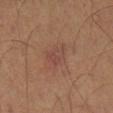The lesion was photographed on a routine skin check and not biopsied; there is no pathology result. About 2.5 mm across. Captured under cross-polarized illumination. A close-up tile cropped from a whole-body skin photograph, about 15 mm across. Automated image analysis of the tile measured a footprint of about 4.5 mm², a shape eccentricity near 0.6, and a shape-asymmetry score of about 0.35 (0 = symmetric). The software also gave peripheral color asymmetry of about 1. Located on the left leg. A male patient, aged approximately 55.